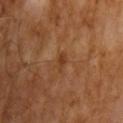follow-up — no biopsy performed (imaged during a skin exam) | lighting — cross-polarized | subject — male, roughly 65 years of age | automated lesion analysis — a lesion area of about 2.5 mm², a shape eccentricity near 0.9, and a shape-asymmetry score of about 0.35 (0 = symmetric); border irregularity of about 3.5 on a 0–10 scale and a color-variation rating of about 1/10 | lesion diameter — ≈2.5 mm | imaging modality — total-body-photography crop, ~15 mm field of view.